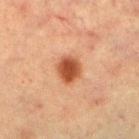diameter: ≈3 mm
image: total-body-photography crop, ~15 mm field of view
location: the left lower leg
tile lighting: cross-polarized illumination
image-analysis metrics: a lesion area of about 7 mm² and a shape-asymmetry score of about 0.15 (0 = symmetric); a lesion color around L≈42 a*≈24 b*≈32 in CIELAB, a lesion–skin lightness drop of about 13, and a lesion-to-skin contrast of about 10.5 (normalized; higher = more distinct); a nevus-likeness score of about 100/100 and lesion-presence confidence of about 100/100
subject: female, in their mid-50s